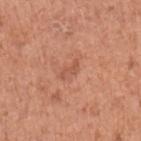The lesion was tiled from a total-body skin photograph and was not biopsied.
The lesion is located on the arm.
A female patient aged approximately 50.
The total-body-photography lesion software estimated a lesion area of about 2.5 mm², a shape eccentricity near 0.9, and two-axis asymmetry of about 0.45. And it measured a mean CIELAB color near L≈54 a*≈27 b*≈32, a lesion–skin lightness drop of about 8, and a normalized border contrast of about 5.5. And it measured a border-irregularity rating of about 5/10, internal color variation of about 0 on a 0–10 scale, and radial color variation of about 0.
This image is a 15 mm lesion crop taken from a total-body photograph.
The tile uses white-light illumination.
Approximately 2.5 mm at its widest.